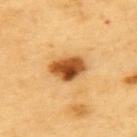notes — catalogued during a skin exam; not biopsied
subject — male, aged 58–62
imaging modality — ~15 mm crop, total-body skin-cancer survey
image-analysis metrics — a footprint of about 9.5 mm², a shape eccentricity near 0.7, and a shape-asymmetry score of about 0.2 (0 = symmetric); a lesion color around L≈53 a*≈26 b*≈45 in CIELAB, a lesion–skin lightness drop of about 20, and a lesion-to-skin contrast of about 12.5 (normalized; higher = more distinct); an automated nevus-likeness rating near 100 out of 100 and lesion-presence confidence of about 100/100
diameter — ~4 mm (longest diameter)
anatomic site — the upper back
lighting — cross-polarized